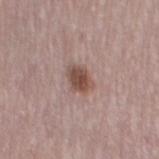Impression: The lesion was tiled from a total-body skin photograph and was not biopsied. Context: Automated image analysis of the tile measured a color-variation rating of about 3.5/10 and radial color variation of about 1. A female subject, aged 48–52. Captured under white-light illumination. A 15 mm close-up extracted from a 3D total-body photography capture. The lesion is on the left thigh.